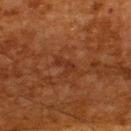Notes:
• follow-up: no biopsy performed (imaged during a skin exam)
• automated lesion analysis: an outline eccentricity of about 0.8 (0 = round, 1 = elongated); an average lesion color of about L≈27 a*≈22 b*≈28 (CIELAB), about 5 CIELAB-L* units darker than the surrounding skin, and a normalized lesion–skin contrast near 5
• lighting: cross-polarized illumination
• anatomic site: the upper back
• lesion size: ~2.5 mm (longest diameter)
• subject: male, aged around 65
• image source: 15 mm crop, total-body photography